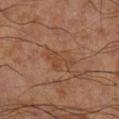No biopsy was performed on this lesion — it was imaged during a full skin examination and was not determined to be concerning. A male subject, about 70 years old. Located on the right lower leg. A 15 mm crop from a total-body photograph taken for skin-cancer surveillance.